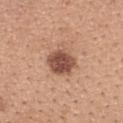Impression:
Part of a total-body skin-imaging series; this lesion was reviewed on a skin check and was not flagged for biopsy.
Context:
The subject is a female aged 28–32. A 15 mm close-up tile from a total-body photography series done for melanoma screening. From the upper back. The tile uses white-light illumination.